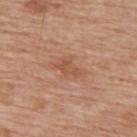About 3.5 mm across.
Captured under white-light illumination.
A 15 mm close-up tile from a total-body photography series done for melanoma screening.
The subject is a male aged 58 to 62.
The lesion is located on the upper back.
The lesion-visualizer software estimated a footprint of about 5 mm², an eccentricity of roughly 0.8, and two-axis asymmetry of about 0.35. The analysis additionally found an automated nevus-likeness rating near 10 out of 100 and a lesion-detection confidence of about 100/100.